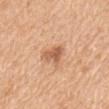Captured during whole-body skin photography for melanoma surveillance; the lesion was not biopsied. The lesion is located on the mid back. The patient is a female aged 53 to 57. Imaged with white-light lighting. Automated tile analysis of the lesion measured a lesion area of about 5.5 mm², an eccentricity of roughly 0.75, and a shape-asymmetry score of about 0.3 (0 = symmetric). The software also gave a lesion-to-skin contrast of about 7.5 (normalized; higher = more distinct). And it measured a border-irregularity index near 3.5/10 and peripheral color asymmetry of about 1. The lesion's longest dimension is about 3 mm. A 15 mm close-up tile from a total-body photography series done for melanoma screening.